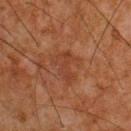{
  "biopsy_status": "not biopsied; imaged during a skin examination",
  "patient": {
    "sex": "male",
    "age_approx": 65
  },
  "image": {
    "source": "total-body photography crop",
    "field_of_view_mm": 15
  },
  "site": "upper back"
}